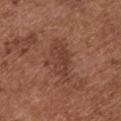Clinical impression: Captured during whole-body skin photography for melanoma surveillance; the lesion was not biopsied. Clinical summary: From the chest. A female subject, in their mid- to late 70s. Longest diameter approximately 5.5 mm. Cropped from a total-body skin-imaging series; the visible field is about 15 mm. Captured under white-light illumination.